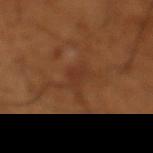Assessment: Imaged during a routine full-body skin examination; the lesion was not biopsied and no histopathology is available. Acquisition and patient details: A close-up tile cropped from a whole-body skin photograph, about 15 mm across. The subject is a male in their mid- to late 60s. Located on the leg. Measured at roughly 2.5 mm in maximum diameter.